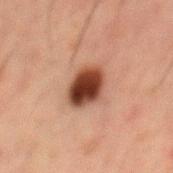Captured during whole-body skin photography for melanoma surveillance; the lesion was not biopsied. The lesion's longest dimension is about 4.5 mm. The lesion is located on the mid back. A male subject, aged around 50. The total-body-photography lesion software estimated a shape eccentricity near 0.65. The software also gave a lesion color around L≈32 a*≈20 b*≈25 in CIELAB, roughly 17 lightness units darker than nearby skin, and a normalized border contrast of about 14. The software also gave a border-irregularity rating of about 1.5/10, a color-variation rating of about 6/10, and radial color variation of about 2. A 15 mm close-up tile from a total-body photography series done for melanoma screening.